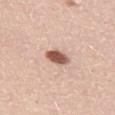Assessment:
Recorded during total-body skin imaging; not selected for excision or biopsy.
Image and clinical context:
A female subject aged approximately 30. An algorithmic analysis of the crop reported a footprint of about 5 mm², an eccentricity of roughly 0.75, and a shape-asymmetry score of about 0.15 (0 = symmetric). It also reported a border-irregularity index near 1.5/10, internal color variation of about 3 on a 0–10 scale, and radial color variation of about 1. It also reported an automated nevus-likeness rating near 100 out of 100 and lesion-presence confidence of about 100/100. On the front of the torso. The lesion's longest dimension is about 3 mm. Imaged with white-light lighting. A 15 mm close-up extracted from a 3D total-body photography capture.